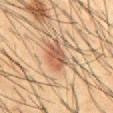Clinical impression: Part of a total-body skin-imaging series; this lesion was reviewed on a skin check and was not flagged for biopsy. Context: Located on the abdomen. The tile uses cross-polarized illumination. A 15 mm close-up extracted from a 3D total-body photography capture. The patient is a male in their mid- to late 30s. The lesion's longest dimension is about 3.5 mm. An algorithmic analysis of the crop reported an area of roughly 7 mm², an outline eccentricity of about 0.75 (0 = round, 1 = elongated), and two-axis asymmetry of about 0.2. It also reported a mean CIELAB color near L≈43 a*≈18 b*≈27 and roughly 9 lightness units darker than nearby skin.